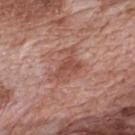Assessment:
Recorded during total-body skin imaging; not selected for excision or biopsy.
Clinical summary:
A male subject, aged 68 to 72. The total-body-photography lesion software estimated a footprint of about 8.5 mm², an eccentricity of roughly 0.8, and two-axis asymmetry of about 0.55. The analysis additionally found a lesion color around L≈50 a*≈24 b*≈27 in CIELAB, roughly 9 lightness units darker than nearby skin, and a normalized border contrast of about 6.5. It also reported a within-lesion color-variation index near 3.5/10 and a peripheral color-asymmetry measure near 1. The analysis additionally found a nevus-likeness score of about 0/100. The lesion is located on the mid back. This image is a 15 mm lesion crop taken from a total-body photograph. The recorded lesion diameter is about 5 mm.